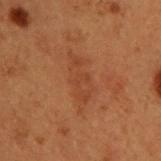This lesion was catalogued during total-body skin photography and was not selected for biopsy. The tile uses cross-polarized illumination. Longest diameter approximately 6 mm. A close-up tile cropped from a whole-body skin photograph, about 15 mm across. Located on the upper back. A male patient about 50 years old.